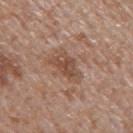Notes:
* follow-up — total-body-photography surveillance lesion; no biopsy
* anatomic site — the mid back
* subject — male, aged approximately 65
* illumination — white-light illumination
* image source — ~15 mm tile from a whole-body skin photo
* image-analysis metrics — a mean CIELAB color near L≈49 a*≈19 b*≈28, a lesion–skin lightness drop of about 9, and a lesion-to-skin contrast of about 7 (normalized; higher = more distinct); a classifier nevus-likeness of about 5/100 and a detector confidence of about 100 out of 100 that the crop contains a lesion